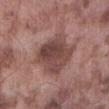Q: Was a biopsy performed?
A: total-body-photography surveillance lesion; no biopsy
Q: What is the imaging modality?
A: ~15 mm tile from a whole-body skin photo
Q: How was the tile lit?
A: white-light illumination
Q: Patient demographics?
A: male, about 75 years old
Q: Where on the body is the lesion?
A: the abdomen
Q: What did automated image analysis measure?
A: a border-irregularity rating of about 2.5/10, a color-variation rating of about 5.5/10, and peripheral color asymmetry of about 2; an automated nevus-likeness rating near 5 out of 100 and a lesion-detection confidence of about 100/100